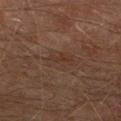Assessment:
The lesion was tiled from a total-body skin photograph and was not biopsied.